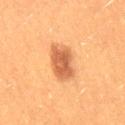biopsy status — imaged on a skin check; not biopsied
illumination — cross-polarized illumination
imaging modality — 15 mm crop, total-body photography
lesion size — about 5 mm
subject — female, aged approximately 40
anatomic site — the right thigh